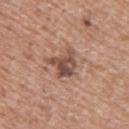No biopsy was performed on this lesion — it was imaged during a full skin examination and was not determined to be concerning. A female subject roughly 40 years of age. An algorithmic analysis of the crop reported an eccentricity of roughly 0.5 and a symmetry-axis asymmetry near 0.45. And it measured border irregularity of about 5 on a 0–10 scale, a within-lesion color-variation index near 6/10, and radial color variation of about 2. The software also gave a nevus-likeness score of about 10/100 and a lesion-detection confidence of about 100/100. A region of skin cropped from a whole-body photographic capture, roughly 15 mm wide. About 4 mm across. The lesion is located on the upper back. The tile uses white-light illumination.